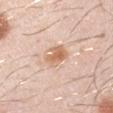The tile uses white-light illumination. A 15 mm close-up extracted from a 3D total-body photography capture. The subject is a male about 30 years old. Approximately 3 mm at its widest. The lesion is located on the left upper arm.